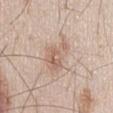This lesion was catalogued during total-body skin photography and was not selected for biopsy. The recorded lesion diameter is about 5 mm. Automated tile analysis of the lesion measured an average lesion color of about L≈62 a*≈17 b*≈27 (CIELAB), roughly 9 lightness units darker than nearby skin, and a normalized lesion–skin contrast near 6. It also reported a border-irregularity index near 5/10, a color-variation rating of about 5/10, and peripheral color asymmetry of about 2. Located on the chest. The tile uses white-light illumination. Cropped from a total-body skin-imaging series; the visible field is about 15 mm. A male subject aged approximately 45.